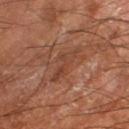image: 15 mm crop, total-body photography
lesion diameter: about 4.5 mm
location: the right lower leg
illumination: cross-polarized
TBP lesion metrics: an area of roughly 6 mm², a shape eccentricity near 0.9, and a shape-asymmetry score of about 0.6 (0 = symmetric); an average lesion color of about L≈40 a*≈22 b*≈29 (CIELAB) and a normalized lesion–skin contrast near 5.5; an automated nevus-likeness rating near 0 out of 100 and a detector confidence of about 90 out of 100 that the crop contains a lesion
subject: male, in their mid- to late 60s